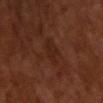Part of a total-body skin-imaging series; this lesion was reviewed on a skin check and was not flagged for biopsy. A male patient roughly 65 years of age. Imaged with cross-polarized lighting. A 15 mm close-up extracted from a 3D total-body photography capture. Approximately 4.5 mm at its widest.